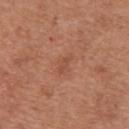Findings:
- notes: imaged on a skin check; not biopsied
- body site: the abdomen
- imaging modality: total-body-photography crop, ~15 mm field of view
- patient: male, aged 63 to 67